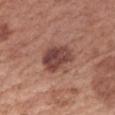Notes:
– follow-up · imaged on a skin check; not biopsied
– site · the right forearm
– patient · female, in their 60s
– illumination · white-light illumination
– imaging modality · 15 mm crop, total-body photography